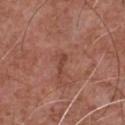Clinical impression: No biopsy was performed on this lesion — it was imaged during a full skin examination and was not determined to be concerning. Background: On the chest. A 15 mm close-up tile from a total-body photography series done for melanoma screening. The patient is a male aged around 75. This is a white-light tile. Automated image analysis of the tile measured border irregularity of about 3.5 on a 0–10 scale and internal color variation of about 0 on a 0–10 scale. The analysis additionally found a classifier nevus-likeness of about 0/100. The recorded lesion diameter is about 2.5 mm.